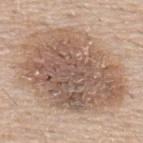Recorded during total-body skin imaging; not selected for excision or biopsy. The patient is a male aged approximately 55. On the back. Cropped from a whole-body photographic skin survey; the tile spans about 15 mm.